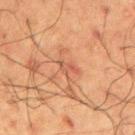The lesion was photographed on a routine skin check and not biopsied; there is no pathology result. Located on the left upper arm. A male patient approximately 60 years of age. A 15 mm close-up tile from a total-body photography series done for melanoma screening.